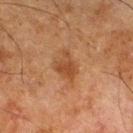workup: imaged on a skin check; not biopsied
image-analysis metrics: a footprint of about 6 mm² and a shape eccentricity near 0.7; border irregularity of about 4 on a 0–10 scale, internal color variation of about 2 on a 0–10 scale, and a peripheral color-asymmetry measure near 1; a classifier nevus-likeness of about 35/100
lighting: cross-polarized
location: the right thigh
acquisition: ~15 mm tile from a whole-body skin photo
subject: male, roughly 80 years of age